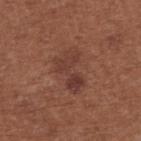<tbp_lesion>
  <biopsy_status>not biopsied; imaged during a skin examination</biopsy_status>
  <lesion_size>
    <long_diameter_mm_approx>4.5</long_diameter_mm_approx>
  </lesion_size>
  <image>
    <source>total-body photography crop</source>
    <field_of_view_mm>15</field_of_view_mm>
  </image>
  <lighting>white-light</lighting>
  <site>upper back</site>
  <patient>
    <sex>female</sex>
    <age_approx>65</age_approx>
  </patient>
</tbp_lesion>